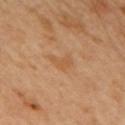The lesion was tiled from a total-body skin photograph and was not biopsied. A lesion tile, about 15 mm wide, cut from a 3D total-body photograph. Automated tile analysis of the lesion measured a footprint of about 3.5 mm². It also reported a lesion color around L≈54 a*≈21 b*≈37 in CIELAB, roughly 6 lightness units darker than nearby skin, and a lesion-to-skin contrast of about 5 (normalized; higher = more distinct). And it measured border irregularity of about 5.5 on a 0–10 scale, internal color variation of about 1 on a 0–10 scale, and a peripheral color-asymmetry measure near 0.5. And it measured an automated nevus-likeness rating near 0 out of 100 and a lesion-detection confidence of about 100/100. This is a cross-polarized tile. The subject is a male in their 50s. The lesion is on the mid back.